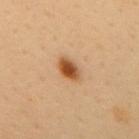The lesion was photographed on a routine skin check and not biopsied; there is no pathology result. A female patient, roughly 50 years of age. On the mid back. This image is a 15 mm lesion crop taken from a total-body photograph. An algorithmic analysis of the crop reported a mean CIELAB color near L≈52 a*≈24 b*≈39 and a normalized border contrast of about 10.5. The recorded lesion diameter is about 3 mm.